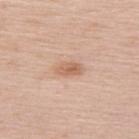Case summary:
- biopsy status: catalogued during a skin exam; not biopsied
- tile lighting: white-light illumination
- acquisition: total-body-photography crop, ~15 mm field of view
- automated lesion analysis: an average lesion color of about L≈62 a*≈20 b*≈31 (CIELAB), about 10 CIELAB-L* units darker than the surrounding skin, and a normalized border contrast of about 6.5
- anatomic site: the back
- lesion diameter: ~3 mm (longest diameter)
- subject: female, approximately 65 years of age